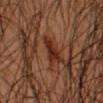Part of a total-body skin-imaging series; this lesion was reviewed on a skin check and was not flagged for biopsy.
The lesion is located on the left forearm.
A 15 mm crop from a total-body photograph taken for skin-cancer surveillance.
The lesion's longest dimension is about 3.5 mm.
An algorithmic analysis of the crop reported a mean CIELAB color near L≈21 a*≈19 b*≈23, about 8 CIELAB-L* units darker than the surrounding skin, and a lesion-to-skin contrast of about 10 (normalized; higher = more distinct). The analysis additionally found a border-irregularity rating of about 4/10 and peripheral color asymmetry of about 1.
The tile uses cross-polarized illumination.
A male subject, about 50 years old.